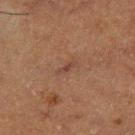Recorded during total-body skin imaging; not selected for excision or biopsy. Captured under cross-polarized illumination. Measured at roughly 3 mm in maximum diameter. On the left thigh. A roughly 15 mm field-of-view crop from a total-body skin photograph. A male patient roughly 75 years of age.